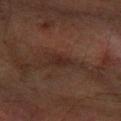notes: imaged on a skin check; not biopsied
lighting: cross-polarized
imaging modality: total-body-photography crop, ~15 mm field of view
lesion size: ≈2.5 mm
anatomic site: the arm
patient: male, roughly 65 years of age